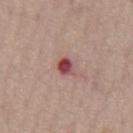From the front of the torso.
Approximately 2.5 mm at its widest.
This is a white-light tile.
Cropped from a whole-body photographic skin survey; the tile spans about 15 mm.
Automated tile analysis of the lesion measured a lesion area of about 4 mm², an outline eccentricity of about 0.6 (0 = round, 1 = elongated), and a shape-asymmetry score of about 0.25 (0 = symmetric). It also reported a mean CIELAB color near L≈48 a*≈28 b*≈21 and a normalized border contrast of about 10. The analysis additionally found a border-irregularity index near 2/10, a within-lesion color-variation index near 9/10, and radial color variation of about 3. And it measured an automated nevus-likeness rating near 0 out of 100 and a detector confidence of about 100 out of 100 that the crop contains a lesion.
A male subject aged around 60.